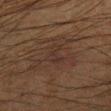follow-up — no biopsy performed (imaged during a skin exam); image — 15 mm crop, total-body photography; illumination — cross-polarized; subject — male, aged approximately 65; location — the left forearm; diameter — about 6.5 mm.